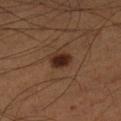workup = total-body-photography surveillance lesion; no biopsy
automated metrics = a classifier nevus-likeness of about 100/100 and a lesion-detection confidence of about 100/100
tile lighting = cross-polarized illumination
subject = male, roughly 55 years of age
image = ~15 mm crop, total-body skin-cancer survey
lesion size = about 2.5 mm
location = the left lower leg